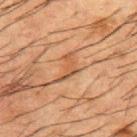location: the upper back | lighting: cross-polarized | patient: male, aged approximately 50 | image: ~15 mm tile from a whole-body skin photo.